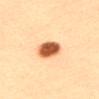This lesion was catalogued during total-body skin photography and was not selected for biopsy. The subject is a female in their mid-30s. Measured at roughly 4.5 mm in maximum diameter. From the upper back. A close-up tile cropped from a whole-body skin photograph, about 15 mm across.